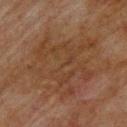A 15 mm close-up tile from a total-body photography series done for melanoma screening.
A male patient, approximately 75 years of age.
The lesion is on the upper back.